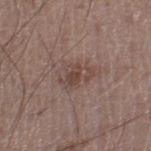Q: Was this lesion biopsied?
A: imaged on a skin check; not biopsied
Q: Patient demographics?
A: male, aged 18 to 22
Q: What did automated image analysis measure?
A: a border-irregularity index near 4.5/10, a within-lesion color-variation index near 4.5/10, and radial color variation of about 1.5; a classifier nevus-likeness of about 0/100
Q: What is the anatomic site?
A: the right lower leg
Q: How was this image acquired?
A: ~15 mm tile from a whole-body skin photo